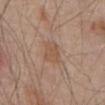| key | value |
|---|---|
| follow-up | imaged on a skin check; not biopsied |
| lesion size | ≈3 mm |
| location | the abdomen |
| TBP lesion metrics | a within-lesion color-variation index near 2.5/10 and peripheral color asymmetry of about 1 |
| image source | total-body-photography crop, ~15 mm field of view |
| lighting | white-light illumination |
| subject | male, aged around 80 |